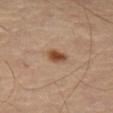No biopsy was performed on this lesion — it was imaged during a full skin examination and was not determined to be concerning.
A female subject aged 58–62.
Imaged with cross-polarized lighting.
The lesion is located on the right leg.
Measured at roughly 2.5 mm in maximum diameter.
A 15 mm close-up tile from a total-body photography series done for melanoma screening.
The lesion-visualizer software estimated a lesion color around L≈49 a*≈21 b*≈33 in CIELAB, roughly 13 lightness units darker than nearby skin, and a normalized lesion–skin contrast near 10. The software also gave a border-irregularity rating of about 2/10, a color-variation rating of about 3.5/10, and peripheral color asymmetry of about 1. And it measured a nevus-likeness score of about 100/100 and a detector confidence of about 100 out of 100 that the crop contains a lesion.